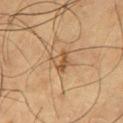Findings:
• biopsy status — no biopsy performed (imaged during a skin exam)
• subject — male, aged approximately 65
• site — the left thigh
• image — ~15 mm crop, total-body skin-cancer survey